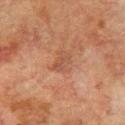notes=imaged on a skin check; not biopsied | subject=male, aged around 75 | TBP lesion metrics=an average lesion color of about L≈42 a*≈20 b*≈27 (CIELAB), a lesion–skin lightness drop of about 6, and a normalized border contrast of about 5; a border-irregularity index near 3/10 and peripheral color asymmetry of about 1; lesion-presence confidence of about 100/100 | image=15 mm crop, total-body photography | location=the left upper arm | lighting=cross-polarized illumination | lesion diameter=about 3 mm.